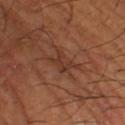Assessment:
Captured during whole-body skin photography for melanoma surveillance; the lesion was not biopsied.
Background:
This is a cross-polarized tile. A male subject, about 65 years old. Measured at roughly 3.5 mm in maximum diameter. On the left lower leg. A close-up tile cropped from a whole-body skin photograph, about 15 mm across. Automated image analysis of the tile measured about 6 CIELAB-L* units darker than the surrounding skin and a lesion-to-skin contrast of about 6 (normalized; higher = more distinct). The software also gave a border-irregularity rating of about 6/10, a color-variation rating of about 1.5/10, and peripheral color asymmetry of about 0.5. The software also gave a nevus-likeness score of about 0/100 and lesion-presence confidence of about 70/100.